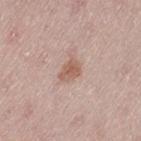workup: imaged on a skin check; not biopsied | anatomic site: the left thigh | lighting: white-light | subject: female, aged around 50 | acquisition: total-body-photography crop, ~15 mm field of view.